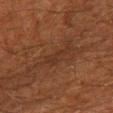{"biopsy_status": "not biopsied; imaged during a skin examination", "patient": {"sex": "male", "age_approx": 60}, "lesion_size": {"long_diameter_mm_approx": 3.5}, "image": {"source": "total-body photography crop", "field_of_view_mm": 15}, "site": "right lower leg"}